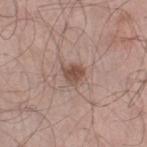{
  "lesion_size": {
    "long_diameter_mm_approx": 2.5
  },
  "patient": {
    "sex": "male",
    "age_approx": 55
  },
  "site": "right lower leg",
  "lighting": "white-light",
  "image": {
    "source": "total-body photography crop",
    "field_of_view_mm": 15
  }
}